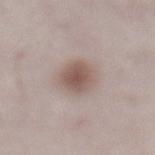Findings:
* patient — male, in their mid- to late 60s
* image — total-body-photography crop, ~15 mm field of view
* automated lesion analysis — a footprint of about 9.5 mm² and two-axis asymmetry of about 0.2; a within-lesion color-variation index near 3.5/10 and radial color variation of about 1; a nevus-likeness score of about 100/100 and a lesion-detection confidence of about 100/100
* location — the abdomen